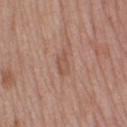biopsy status = catalogued during a skin exam; not biopsied
site = the right thigh
subject = female, in their 50s
acquisition = ~15 mm tile from a whole-body skin photo
diameter = about 3 mm